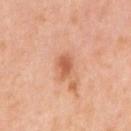follow-up = total-body-photography surveillance lesion; no biopsy
lesion diameter = ≈2.5 mm
acquisition = ~15 mm tile from a whole-body skin photo
illumination = white-light illumination
body site = the arm
subject = female, about 40 years old